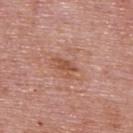biopsy_status: not biopsied; imaged during a skin examination
lesion_size:
  long_diameter_mm_approx: 2.5
lighting: white-light
site: upper back
patient:
  sex: male
  age_approx: 75
image:
  source: total-body photography crop
  field_of_view_mm: 15